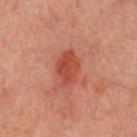follow-up: catalogued during a skin exam; not biopsied
acquisition: 15 mm crop, total-body photography
lesion diameter: ≈4 mm
anatomic site: the left upper arm
TBP lesion metrics: an outline eccentricity of about 0.75 (0 = round, 1 = elongated) and a shape-asymmetry score of about 0.15 (0 = symmetric); a lesion color around L≈47 a*≈31 b*≈33 in CIELAB, a lesion–skin lightness drop of about 10, and a normalized border contrast of about 7.5; a border-irregularity index near 1.5/10 and internal color variation of about 2.5 on a 0–10 scale
patient: male, aged approximately 65